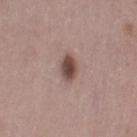{
  "biopsy_status": "not biopsied; imaged during a skin examination",
  "lesion_size": {
    "long_diameter_mm_approx": 3.0
  },
  "lighting": "white-light",
  "patient": {
    "sex": "female",
    "age_approx": 50
  },
  "image": {
    "source": "total-body photography crop",
    "field_of_view_mm": 15
  },
  "site": "leg"
}